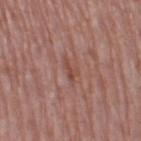Clinical impression:
The lesion was tiled from a total-body skin photograph and was not biopsied.
Acquisition and patient details:
Captured under white-light illumination. An algorithmic analysis of the crop reported a shape eccentricity near 0.85 and a symmetry-axis asymmetry near 0.55. And it measured an automated nevus-likeness rating near 0 out of 100 and lesion-presence confidence of about 90/100. Longest diameter approximately 2.5 mm. This image is a 15 mm lesion crop taken from a total-body photograph. The patient is a female aged approximately 60. Located on the left thigh.